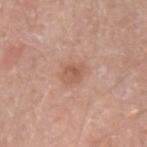<lesion>
  <biopsy_status>not biopsied; imaged during a skin examination</biopsy_status>
  <image>
    <source>total-body photography crop</source>
    <field_of_view_mm>15</field_of_view_mm>
  </image>
  <site>left forearm</site>
  <lesion_size>
    <long_diameter_mm_approx>3.0</long_diameter_mm_approx>
  </lesion_size>
  <lighting>white-light</lighting>
  <patient>
    <sex>male</sex>
    <age_approx>50</age_approx>
  </patient>
</lesion>